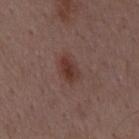{
  "biopsy_status": "not biopsied; imaged during a skin examination",
  "image": {
    "source": "total-body photography crop",
    "field_of_view_mm": 15
  },
  "site": "mid back",
  "patient": {
    "sex": "male",
    "age_approx": 50
  }
}